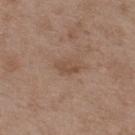follow-up: total-body-photography surveillance lesion; no biopsy | location: the upper back | patient: male, aged 48–52 | image source: total-body-photography crop, ~15 mm field of view.